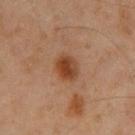Part of a total-body skin-imaging series; this lesion was reviewed on a skin check and was not flagged for biopsy. A 15 mm close-up extracted from a 3D total-body photography capture. A male subject, approximately 60 years of age. On the front of the torso. Measured at roughly 3.5 mm in maximum diameter. The tile uses cross-polarized illumination.